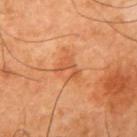notes — no biopsy performed (imaged during a skin exam) | tile lighting — cross-polarized | body site — the right upper arm | image source — ~15 mm tile from a whole-body skin photo | image-analysis metrics — a footprint of about 6 mm², a shape eccentricity near 0.7, and a symmetry-axis asymmetry near 0.45; a border-irregularity rating of about 6/10, a within-lesion color-variation index near 2.5/10, and a peripheral color-asymmetry measure near 1; a nevus-likeness score of about 5/100 and a lesion-detection confidence of about 100/100 | patient — male, aged approximately 65 | diameter — ≈3.5 mm.